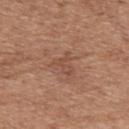• image-analysis metrics — an area of roughly 3.5 mm² and a shape-asymmetry score of about 0.55 (0 = symmetric); a border-irregularity rating of about 6.5/10 and a color-variation rating of about 0/10; an automated nevus-likeness rating near 0 out of 100 and a lesion-detection confidence of about 100/100
• location — the back
• subject — male, aged 68 to 72
• diameter — ~3 mm (longest diameter)
• acquisition — 15 mm crop, total-body photography